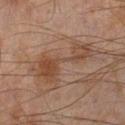  automated_metrics:
    cielab_L: 36
    cielab_a: 16
    cielab_b: 24
    vs_skin_darker_L: 7.0
    vs_skin_contrast_norm: 7.0
  image:
    source: total-body photography crop
    field_of_view_mm: 15
  lighting: cross-polarized
  lesion_size:
    long_diameter_mm_approx: 8.0
  patient:
    sex: male
    age_approx: 45
  site: left lower leg